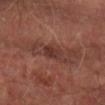Notes:
• notes: imaged on a skin check; not biopsied
• anatomic site: the arm
• patient: male, aged around 65
• imaging modality: ~15 mm tile from a whole-body skin photo